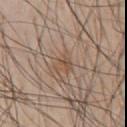{
  "image": {
    "source": "total-body photography crop",
    "field_of_view_mm": 15
  },
  "site": "front of the torso",
  "patient": {
    "sex": "male",
    "age_approx": 60
  },
  "lesion_size": {
    "long_diameter_mm_approx": 3.0
  },
  "automated_metrics": {
    "area_mm2_approx": 4.5,
    "eccentricity": 0.5,
    "shape_asymmetry": 0.4,
    "nevus_likeness_0_100": 0,
    "lesion_detection_confidence_0_100": 100
  }
}